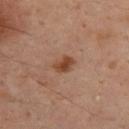Part of a total-body skin-imaging series; this lesion was reviewed on a skin check and was not flagged for biopsy.
The patient is a male roughly 50 years of age.
This is a cross-polarized tile.
About 2.5 mm across.
This image is a 15 mm lesion crop taken from a total-body photograph.
On the upper back.